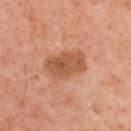Q: Patient demographics?
A: male, aged 53 to 57
Q: What kind of image is this?
A: ~15 mm crop, total-body skin-cancer survey
Q: Lesion size?
A: about 5 mm
Q: What did automated image analysis measure?
A: an area of roughly 13 mm², an eccentricity of roughly 0.75, and two-axis asymmetry of about 0.15; a lesion color around L≈55 a*≈24 b*≈37 in CIELAB, roughly 10 lightness units darker than nearby skin, and a lesion-to-skin contrast of about 7.5 (normalized; higher = more distinct); a nevus-likeness score of about 20/100 and lesion-presence confidence of about 100/100
Q: Lesion location?
A: the upper back
Q: How was the tile lit?
A: cross-polarized